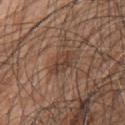Recorded during total-body skin imaging; not selected for excision or biopsy.
Cropped from a whole-body photographic skin survey; the tile spans about 15 mm.
Imaged with white-light lighting.
The recorded lesion diameter is about 4 mm.
A male subject aged 48 to 52.
The lesion is located on the upper back.